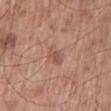  lighting: white-light
  lesion_size:
    long_diameter_mm_approx: 3.0
  image:
    source: total-body photography crop
    field_of_view_mm: 15
  site: mid back
  patient:
    sex: male
    age_approx: 55